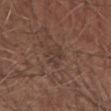No biopsy was performed on this lesion — it was imaged during a full skin examination and was not determined to be concerning. On the right upper arm. The total-body-photography lesion software estimated a lesion–skin lightness drop of about 5. It also reported a color-variation rating of about 2/10. It also reported a classifier nevus-likeness of about 0/100 and a detector confidence of about 70 out of 100 that the crop contains a lesion. A male subject, roughly 75 years of age. A region of skin cropped from a whole-body photographic capture, roughly 15 mm wide.